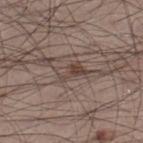follow-up — total-body-photography surveillance lesion; no biopsy | illumination — white-light | image source — total-body-photography crop, ~15 mm field of view | size — ≈3.5 mm | body site — the right lower leg | subject — male, aged around 30.